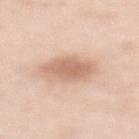Part of a total-body skin-imaging series; this lesion was reviewed on a skin check and was not flagged for biopsy. A female subject, aged around 50. Captured under white-light illumination. A lesion tile, about 15 mm wide, cut from a 3D total-body photograph. From the back.